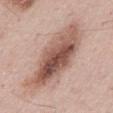The lesion was photographed on a routine skin check and not biopsied; there is no pathology result.
Longest diameter approximately 9.5 mm.
On the mid back.
The patient is a male aged 53–57.
Automated tile analysis of the lesion measured a shape eccentricity near 0.9 and a shape-asymmetry score of about 0.2 (0 = symmetric). And it measured a lesion color around L≈55 a*≈20 b*≈25 in CIELAB, a lesion–skin lightness drop of about 15, and a lesion-to-skin contrast of about 10 (normalized; higher = more distinct). And it measured border irregularity of about 3 on a 0–10 scale and radial color variation of about 3.
The tile uses white-light illumination.
A 15 mm crop from a total-body photograph taken for skin-cancer surveillance.